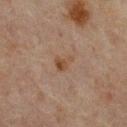follow-up = no biopsy performed (imaged during a skin exam)
patient = male, aged approximately 65
lighting = cross-polarized
lesion size = about 2.5 mm
automated lesion analysis = a mean CIELAB color near L≈37 a*≈15 b*≈25, roughly 6 lightness units darker than nearby skin, and a normalized border contrast of about 7; a classifier nevus-likeness of about 10/100 and a lesion-detection confidence of about 100/100
anatomic site = the back
image source = 15 mm crop, total-body photography